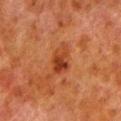– follow-up · no biopsy performed (imaged during a skin exam)
– imaging modality · ~15 mm tile from a whole-body skin photo
– location · the left lower leg
– patient · male, aged around 80